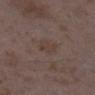Q: What is the lesion's diameter?
A: ≈2.5 mm
Q: Who is the patient?
A: female, about 35 years old
Q: Lesion location?
A: the right lower leg
Q: What kind of image is this?
A: total-body-photography crop, ~15 mm field of view
Q: Automated lesion metrics?
A: a footprint of about 5 mm², an outline eccentricity of about 0.5 (0 = round, 1 = elongated), and a symmetry-axis asymmetry near 0.2; a mean CIELAB color near L≈39 a*≈13 b*≈21, a lesion–skin lightness drop of about 5, and a lesion-to-skin contrast of about 5 (normalized; higher = more distinct); internal color variation of about 1 on a 0–10 scale; an automated nevus-likeness rating near 0 out of 100 and a detector confidence of about 100 out of 100 that the crop contains a lesion
Q: What lighting was used for the tile?
A: white-light